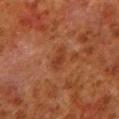| feature | finding |
|---|---|
| biopsy status | total-body-photography surveillance lesion; no biopsy |
| lighting | cross-polarized illumination |
| size | about 2.5 mm |
| imaging modality | ~15 mm crop, total-body skin-cancer survey |
| anatomic site | the left lower leg |
| subject | male, about 80 years old |